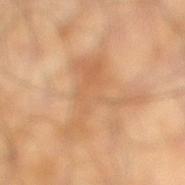{"biopsy_status": "not biopsied; imaged during a skin examination", "patient": {"sex": "male", "age_approx": 65}, "site": "right lower leg", "lesion_size": {"long_diameter_mm_approx": 8.5}, "lighting": "cross-polarized", "image": {"source": "total-body photography crop", "field_of_view_mm": 15}}